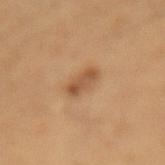  biopsy_status: not biopsied; imaged during a skin examination
  patient:
    sex: male
    age_approx: 60
  image:
    source: total-body photography crop
    field_of_view_mm: 15
  site: lower back